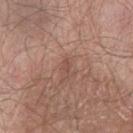An algorithmic analysis of the crop reported a classifier nevus-likeness of about 0/100 and lesion-presence confidence of about 100/100.
The patient is a male aged approximately 65.
A 15 mm close-up tile from a total-body photography series done for melanoma screening.
Located on the left thigh.